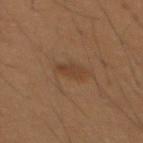No biopsy was performed on this lesion — it was imaged during a full skin examination and was not determined to be concerning.
Measured at roughly 2.5 mm in maximum diameter.
This is a cross-polarized tile.
This image is a 15 mm lesion crop taken from a total-body photograph.
From the abdomen.
An algorithmic analysis of the crop reported a lesion area of about 4 mm² and an eccentricity of roughly 0.7. It also reported a mean CIELAB color near L≈31 a*≈15 b*≈25. The analysis additionally found a border-irregularity index near 2/10, a color-variation rating of about 2/10, and a peripheral color-asymmetry measure near 0.5. The software also gave lesion-presence confidence of about 100/100.
A male subject aged 48–52.